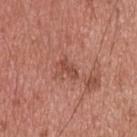Clinical impression: Captured during whole-body skin photography for melanoma surveillance; the lesion was not biopsied. Background: Automated tile analysis of the lesion measured a nevus-likeness score of about 5/100 and lesion-presence confidence of about 100/100. A male subject, in their mid- to late 50s. From the back. The tile uses white-light illumination. About 2.5 mm across. A 15 mm crop from a total-body photograph taken for skin-cancer surveillance.